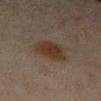{
  "biopsy_status": "not biopsied; imaged during a skin examination",
  "lighting": "cross-polarized",
  "lesion_size": {
    "long_diameter_mm_approx": 3.5
  },
  "image": {
    "source": "total-body photography crop",
    "field_of_view_mm": 15
  },
  "site": "leg",
  "automated_metrics": {
    "cielab_L": 28,
    "cielab_a": 13,
    "cielab_b": 22,
    "vs_skin_darker_L": 8.0,
    "vs_skin_contrast_norm": 9.0,
    "border_irregularity_0_10": 2.5,
    "color_variation_0_10": 3.0,
    "peripheral_color_asymmetry": 1.0
  },
  "patient": {
    "sex": "female",
    "age_approx": 45
  }
}